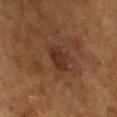The lesion was tiled from a total-body skin photograph and was not biopsied. The lesion is on the chest. This is a cross-polarized tile. The subject is a male roughly 60 years of age. Approximately 3.5 mm at its widest. Cropped from a whole-body photographic skin survey; the tile spans about 15 mm.